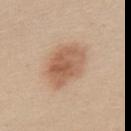| field | value |
|---|---|
| workup | imaged on a skin check; not biopsied |
| location | the mid back |
| lesion size | about 5 mm |
| image | ~15 mm tile from a whole-body skin photo |
| tile lighting | white-light illumination |
| subject | female, aged 18 to 22 |